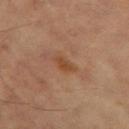- biopsy status — imaged on a skin check; not biopsied